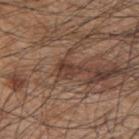lesion diameter: ≈3 mm
acquisition: ~15 mm tile from a whole-body skin photo
illumination: white-light illumination
subject: male, in their mid- to late 40s
site: the upper back